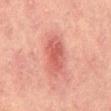Notes:
– follow-up: imaged on a skin check; not biopsied
– acquisition: ~15 mm tile from a whole-body skin photo
– lesion size: ≈5 mm
– lighting: cross-polarized
– location: the back
– image-analysis metrics: a lesion–skin lightness drop of about 9 and a normalized lesion–skin contrast near 6.5; a classifier nevus-likeness of about 65/100 and lesion-presence confidence of about 100/100
– patient: male, aged approximately 45